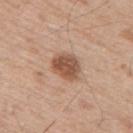Case summary:
• follow-up — catalogued during a skin exam; not biopsied
• anatomic site — the upper back
• image source — 15 mm crop, total-body photography
• subject — male, approximately 55 years of age
• diameter — ≈3.5 mm